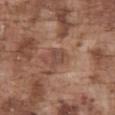The lesion was tiled from a total-body skin photograph and was not biopsied. A male patient aged 73 to 77. Imaged with white-light lighting. Automated tile analysis of the lesion measured a lesion area of about 3.5 mm², an outline eccentricity of about 0.65 (0 = round, 1 = elongated), and a shape-asymmetry score of about 0.3 (0 = symmetric). It also reported an average lesion color of about L≈46 a*≈20 b*≈25 (CIELAB) and a lesion-to-skin contrast of about 6 (normalized; higher = more distinct). And it measured an automated nevus-likeness rating near 5 out of 100 and a detector confidence of about 100 out of 100 that the crop contains a lesion. A lesion tile, about 15 mm wide, cut from a 3D total-body photograph. From the front of the torso.